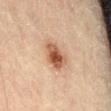| field | value |
|---|---|
| notes | no biopsy performed (imaged during a skin exam) |
| image source | 15 mm crop, total-body photography |
| patient | male, aged 43–47 |
| location | the abdomen |